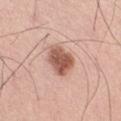Assessment: Imaged during a routine full-body skin examination; the lesion was not biopsied and no histopathology is available. Acquisition and patient details: This is a white-light tile. The lesion is on the right thigh. Measured at roughly 4 mm in maximum diameter. A male patient, approximately 70 years of age. This image is a 15 mm lesion crop taken from a total-body photograph.